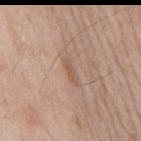Part of a total-body skin-imaging series; this lesion was reviewed on a skin check and was not flagged for biopsy.
A male subject, aged 63 to 67.
A close-up tile cropped from a whole-body skin photograph, about 15 mm across.
The lesion's longest dimension is about 3 mm.
On the mid back.